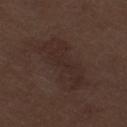The lesion was photographed on a routine skin check and not biopsied; there is no pathology result. A male subject aged around 70. The lesion is on the leg. Cropped from a whole-body photographic skin survey; the tile spans about 15 mm. Imaged with white-light lighting. The recorded lesion diameter is about 7.5 mm.